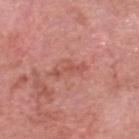<lesion>
  <biopsy_status>not biopsied; imaged during a skin examination</biopsy_status>
  <lighting>white-light</lighting>
  <site>head or neck</site>
  <patient>
    <sex>male</sex>
    <age_approx>70</age_approx>
  </patient>
  <image>
    <source>total-body photography crop</source>
    <field_of_view_mm>15</field_of_view_mm>
  </image>
  <lesion_size>
    <long_diameter_mm_approx>3.5</long_diameter_mm_approx>
  </lesion_size>
</lesion>